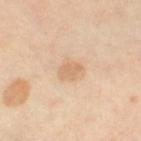Recorded during total-body skin imaging; not selected for excision or biopsy.
A 15 mm close-up tile from a total-body photography series done for melanoma screening.
Located on the leg.
Measured at roughly 2.5 mm in maximum diameter.
The tile uses cross-polarized illumination.
A female subject aged 48–52.